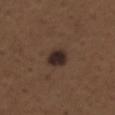Part of a total-body skin-imaging series; this lesion was reviewed on a skin check and was not flagged for biopsy. The lesion is on the upper back. Imaged with white-light lighting. A male subject aged approximately 70. A close-up tile cropped from a whole-body skin photograph, about 15 mm across. The lesion-visualizer software estimated an area of roughly 6 mm² and an outline eccentricity of about 0.65 (0 = round, 1 = elongated). The software also gave an average lesion color of about L≈27 a*≈13 b*≈19 (CIELAB) and about 12 CIELAB-L* units darker than the surrounding skin. The software also gave a border-irregularity rating of about 1/10. The analysis additionally found a nevus-likeness score of about 85/100.